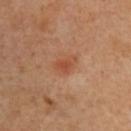Captured during whole-body skin photography for melanoma surveillance; the lesion was not biopsied. Automated image analysis of the tile measured an eccentricity of roughly 0.75 and a shape-asymmetry score of about 0.3 (0 = symmetric). The analysis additionally found border irregularity of about 3 on a 0–10 scale, a within-lesion color-variation index near 2.5/10, and peripheral color asymmetry of about 0.5. Measured at roughly 3 mm in maximum diameter. Captured under cross-polarized illumination. The lesion is on the chest. The subject is a male approximately 50 years of age. A 15 mm close-up tile from a total-body photography series done for melanoma screening.